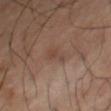<case>
  <biopsy_status>not biopsied; imaged during a skin examination</biopsy_status>
  <lesion_size>
    <long_diameter_mm_approx>2.5</long_diameter_mm_approx>
  </lesion_size>
  <patient>
    <sex>male</sex>
    <age_approx>65</age_approx>
  </patient>
  <image>
    <source>total-body photography crop</source>
    <field_of_view_mm>15</field_of_view_mm>
  </image>
  <site>right thigh</site>
  <automated_metrics>
    <eccentricity>0.85</eccentricity>
    <shape_asymmetry>0.4</shape_asymmetry>
    <cielab_L>43</cielab_L>
    <cielab_a>17</cielab_a>
    <cielab_b>26</cielab_b>
    <vs_skin_darker_L>6.0</vs_skin_darker_L>
    <color_variation_0_10>1.0</color_variation_0_10>
    <peripheral_color_asymmetry>0.5</peripheral_color_asymmetry>
  </automated_metrics>
  <lighting>cross-polarized</lighting>
</case>